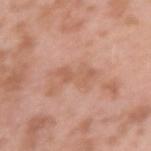Clinical impression:
The lesion was tiled from a total-body skin photograph and was not biopsied.
Clinical summary:
A 15 mm close-up extracted from a 3D total-body photography capture. This is a white-light tile. An algorithmic analysis of the crop reported a lesion area of about 5.5 mm², a shape eccentricity near 0.95, and a shape-asymmetry score of about 0.3 (0 = symmetric). It also reported border irregularity of about 5 on a 0–10 scale, internal color variation of about 2 on a 0–10 scale, and peripheral color asymmetry of about 0.5. The lesion is located on the left upper arm. A male subject, aged approximately 40.